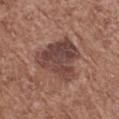Case summary:
• follow-up — catalogued during a skin exam; not biopsied
• imaging modality — 15 mm crop, total-body photography
• patient — female, roughly 75 years of age
• body site — the leg